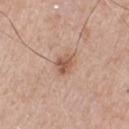biopsy status: total-body-photography surveillance lesion; no biopsy | lighting: white-light illumination | subject: male, aged approximately 80 | image: ~15 mm tile from a whole-body skin photo | body site: the front of the torso.